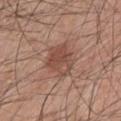Assessment:
Recorded during total-body skin imaging; not selected for excision or biopsy.
Background:
A 15 mm close-up tile from a total-body photography series done for melanoma screening. From the left upper arm. The recorded lesion diameter is about 4 mm. The patient is a male approximately 45 years of age. Automated image analysis of the tile measured a footprint of about 11 mm² and an eccentricity of roughly 0.5. The software also gave a lesion color around L≈48 a*≈21 b*≈26 in CIELAB, a lesion–skin lightness drop of about 9, and a normalized border contrast of about 7. It also reported a border-irregularity index near 3/10 and a within-lesion color-variation index near 4.5/10. It also reported a nevus-likeness score of about 70/100 and lesion-presence confidence of about 100/100. Imaged with white-light lighting.